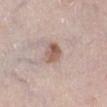follow-up = catalogued during a skin exam; not biopsied
imaging modality = 15 mm crop, total-body photography
body site = the left lower leg
subject = female, aged approximately 65